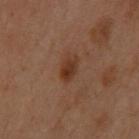No biopsy was performed on this lesion — it was imaged during a full skin examination and was not determined to be concerning. Measured at roughly 3 mm in maximum diameter. A close-up tile cropped from a whole-body skin photograph, about 15 mm across. The lesion is located on the upper back. Imaged with cross-polarized lighting. The subject is a female aged 53 to 57.